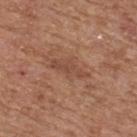Imaged during a routine full-body skin examination; the lesion was not biopsied and no histopathology is available.
Approximately 4.5 mm at its widest.
Automated tile analysis of the lesion measured an area of roughly 6.5 mm² and an outline eccentricity of about 0.9 (0 = round, 1 = elongated). The analysis additionally found a mean CIELAB color near L≈47 a*≈22 b*≈29, roughly 7 lightness units darker than nearby skin, and a normalized border contrast of about 5.5. The analysis additionally found lesion-presence confidence of about 95/100.
A male patient, in their mid-60s.
The tile uses white-light illumination.
A 15 mm close-up tile from a total-body photography series done for melanoma screening.
The lesion is located on the back.